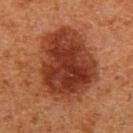The lesion was photographed on a routine skin check and not biopsied; there is no pathology result. The lesion is located on the mid back. Longest diameter approximately 9 mm. Cropped from a whole-body photographic skin survey; the tile spans about 15 mm. The subject is a male approximately 80 years of age. Imaged with cross-polarized lighting.